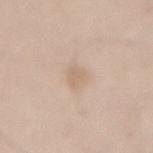An algorithmic analysis of the crop reported a footprint of about 4.5 mm², an outline eccentricity of about 0.75 (0 = round, 1 = elongated), and a shape-asymmetry score of about 0.3 (0 = symmetric). It also reported an average lesion color of about L≈66 a*≈14 b*≈29 (CIELAB) and a normalized border contrast of about 4.5. The analysis additionally found a border-irregularity index near 2.5/10, internal color variation of about 1 on a 0–10 scale, and a peripheral color-asymmetry measure near 0.5.
A region of skin cropped from a whole-body photographic capture, roughly 15 mm wide.
Approximately 3 mm at its widest.
The tile uses white-light illumination.
A male patient in their 50s.
The lesion is located on the lower back.